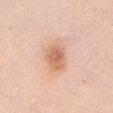The lesion was photographed on a routine skin check and not biopsied; there is no pathology result.
Located on the chest.
A female subject, in their 50s.
A close-up tile cropped from a whole-body skin photograph, about 15 mm across.